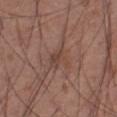<tbp_lesion>
<biopsy_status>not biopsied; imaged during a skin examination</biopsy_status>
<site>front of the torso</site>
<lighting>white-light</lighting>
<image>
  <source>total-body photography crop</source>
  <field_of_view_mm>15</field_of_view_mm>
</image>
<lesion_size>
  <long_diameter_mm_approx>3.0</long_diameter_mm_approx>
</lesion_size>
<automated_metrics>
  <area_mm2_approx>5.5</area_mm2_approx>
  <eccentricity>0.45</eccentricity>
  <shape_asymmetry>0.35</shape_asymmetry>
  <cielab_L>44</cielab_L>
  <cielab_a>19</cielab_a>
  <cielab_b>25</cielab_b>
  <vs_skin_darker_L>6.0</vs_skin_darker_L>
  <vs_skin_contrast_norm>5.0</vs_skin_contrast_norm>
  <border_irregularity_0_10>3.5</border_irregularity_0_10>
  <peripheral_color_asymmetry>1.0</peripheral_color_asymmetry>
  <nevus_likeness_0_100>0</nevus_likeness_0_100>
  <lesion_detection_confidence_0_100>90</lesion_detection_confidence_0_100>
</automated_metrics>
<patient>
  <sex>male</sex>
  <age_approx>55</age_approx>
</patient>
</tbp_lesion>